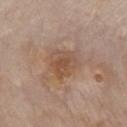| feature | finding |
|---|---|
| acquisition | total-body-photography crop, ~15 mm field of view |
| subject | female, aged 63 to 67 |
| diameter | ~4 mm (longest diameter) |
| site | the chest |
| illumination | white-light illumination |
| automated lesion analysis | border irregularity of about 2 on a 0–10 scale, a color-variation rating of about 3.5/10, and a peripheral color-asymmetry measure near 1 |